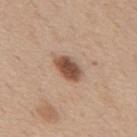Assessment:
The lesion was tiled from a total-body skin photograph and was not biopsied.
Background:
A male subject, in their 60s. The lesion-visualizer software estimated a lesion color around L≈51 a*≈20 b*≈29 in CIELAB, about 15 CIELAB-L* units darker than the surrounding skin, and a lesion-to-skin contrast of about 10.5 (normalized; higher = more distinct). And it measured a border-irregularity index near 1.5/10, a within-lesion color-variation index near 4/10, and radial color variation of about 1. The analysis additionally found an automated nevus-likeness rating near 95 out of 100 and a lesion-detection confidence of about 100/100. From the mid back. Measured at roughly 4 mm in maximum diameter. A 15 mm close-up tile from a total-body photography series done for melanoma screening.